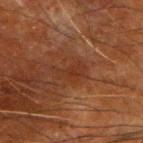Captured during whole-body skin photography for melanoma surveillance; the lesion was not biopsied.
Located on the right forearm.
Approximately 4 mm at its widest.
A 15 mm crop from a total-body photograph taken for skin-cancer surveillance.
Automated image analysis of the tile measured a mean CIELAB color near L≈27 a*≈20 b*≈27, about 5 CIELAB-L* units darker than the surrounding skin, and a lesion-to-skin contrast of about 5.5 (normalized; higher = more distinct). And it measured border irregularity of about 5 on a 0–10 scale, a color-variation rating of about 2/10, and peripheral color asymmetry of about 0.5. The analysis additionally found a nevus-likeness score of about 0/100 and a lesion-detection confidence of about 95/100.
This is a cross-polarized tile.
A male patient aged around 65.